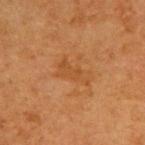biopsy status = total-body-photography surveillance lesion; no biopsy
size = ≈4 mm
patient = female, in their 40s
tile lighting = cross-polarized
acquisition = ~15 mm crop, total-body skin-cancer survey
site = the back